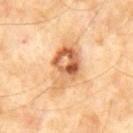- workup — catalogued during a skin exam; not biopsied
- image — ~15 mm tile from a whole-body skin photo
- subject — male, about 60 years old
- body site — the abdomen
- tile lighting — cross-polarized
- lesion diameter — ~6 mm (longest diameter)